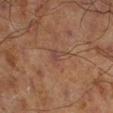Part of a total-body skin-imaging series; this lesion was reviewed on a skin check and was not flagged for biopsy.
Longest diameter approximately 2.5 mm.
This image is a 15 mm lesion crop taken from a total-body photograph.
From the right lower leg.
Captured under cross-polarized illumination.
A male subject aged 68–72.